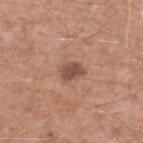biopsy status — no biopsy performed (imaged during a skin exam) | patient — male, aged 48–52 | tile lighting — white-light illumination | lesion diameter — ~2.5 mm (longest diameter) | imaging modality — ~15 mm crop, total-body skin-cancer survey | automated metrics — a border-irregularity rating of about 2.5/10 and a within-lesion color-variation index near 2/10; a lesion-detection confidence of about 100/100 | body site — the left lower leg.